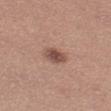Imaged during a routine full-body skin examination; the lesion was not biopsied and no histopathology is available. Automated tile analysis of the lesion measured a lesion color around L≈50 a*≈20 b*≈25 in CIELAB and a normalized lesion–skin contrast near 8.5. It also reported border irregularity of about 1.5 on a 0–10 scale, internal color variation of about 4 on a 0–10 scale, and peripheral color asymmetry of about 1. It also reported a nevus-likeness score of about 90/100. From the left thigh. A female subject aged approximately 30. This is a white-light tile. Cropped from a total-body skin-imaging series; the visible field is about 15 mm. About 3 mm across.